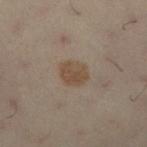workup = no biopsy performed (imaged during a skin exam)
image source = ~15 mm tile from a whole-body skin photo
subject = female, aged approximately 30
site = the left leg